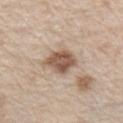follow-up=catalogued during a skin exam; not biopsied | lighting=white-light | body site=the chest | subject=male, roughly 80 years of age | lesion diameter=≈4 mm | acquisition=~15 mm crop, total-body skin-cancer survey.